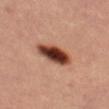A female subject roughly 45 years of age.
Measured at roughly 5.5 mm in maximum diameter.
This image is a 15 mm lesion crop taken from a total-body photograph.
The tile uses cross-polarized illumination.
From the left thigh.